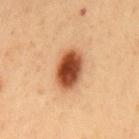Recorded during total-body skin imaging; not selected for excision or biopsy. The lesion's longest dimension is about 5 mm. Located on the mid back. This is a cross-polarized tile. A 15 mm close-up tile from a total-body photography series done for melanoma screening. The patient is a male roughly 50 years of age.